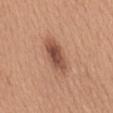– follow-up: no biopsy performed (imaged during a skin exam)
– image: ~15 mm crop, total-body skin-cancer survey
– subject: female, roughly 55 years of age
– lighting: white-light illumination
– site: the back
– image-analysis metrics: a border-irregularity index near 1.5/10 and radial color variation of about 1.5; a nevus-likeness score of about 75/100
– size: about 5.5 mm